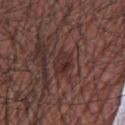Notes:
- biopsy status · no biopsy performed (imaged during a skin exam)
- image · ~15 mm tile from a whole-body skin photo
- subject · male, aged approximately 75
- anatomic site · the front of the torso